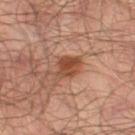| field | value |
|---|---|
| biopsy status | total-body-photography surveillance lesion; no biopsy |
| location | the left thigh |
| image | total-body-photography crop, ~15 mm field of view |
| lesion size | ≈4 mm |
| patient | male, in their mid- to late 60s |